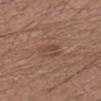The lesion's longest dimension is about 2.5 mm. This is a white-light tile. An algorithmic analysis of the crop reported a footprint of about 4 mm² and a symmetry-axis asymmetry near 0.45. And it measured a border-irregularity index near 4.5/10, a color-variation rating of about 2/10, and a peripheral color-asymmetry measure near 1. The lesion is located on the left forearm. This image is a 15 mm lesion crop taken from a total-body photograph. A male subject roughly 40 years of age.